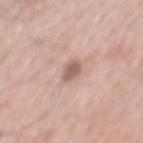follow-up = no biopsy performed (imaged during a skin exam)
acquisition = ~15 mm crop, total-body skin-cancer survey
site = the mid back
tile lighting = white-light
image-analysis metrics = a mean CIELAB color near L≈59 a*≈19 b*≈25, roughly 12 lightness units darker than nearby skin, and a normalized lesion–skin contrast near 7.5
size = about 2.5 mm
subject = male, aged 58–62